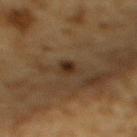Impression:
Part of a total-body skin-imaging series; this lesion was reviewed on a skin check and was not flagged for biopsy.
Acquisition and patient details:
A male subject, aged 83 or over. Cropped from a whole-body photographic skin survey; the tile spans about 15 mm. Located on the upper back. About 2.5 mm across. An algorithmic analysis of the crop reported a lesion-detection confidence of about 100/100. Captured under cross-polarized illumination.